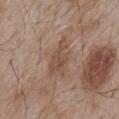Captured during whole-body skin photography for melanoma surveillance; the lesion was not biopsied. The lesion is on the mid back. Automated image analysis of the tile measured a footprint of about 9 mm², an outline eccentricity of about 0.9 (0 = round, 1 = elongated), and two-axis asymmetry of about 0.45. The analysis additionally found a border-irregularity rating of about 5.5/10 and a peripheral color-asymmetry measure near 1. And it measured a classifier nevus-likeness of about 0/100 and a detector confidence of about 100 out of 100 that the crop contains a lesion. Imaged with white-light lighting. A male patient, aged 53 to 57. A 15 mm close-up extracted from a 3D total-body photography capture.